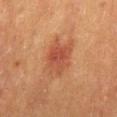| key | value |
|---|---|
| follow-up | catalogued during a skin exam; not biopsied |
| lighting | cross-polarized illumination |
| anatomic site | the mid back |
| patient | male, aged around 50 |
| image-analysis metrics | a footprint of about 14 mm², an eccentricity of roughly 0.75, and a shape-asymmetry score of about 0.25 (0 = symmetric); a border-irregularity index near 3/10, a color-variation rating of about 5/10, and peripheral color asymmetry of about 1.5; a nevus-likeness score of about 45/100 and a lesion-detection confidence of about 100/100 |
| size | ≈5 mm |
| acquisition | ~15 mm crop, total-body skin-cancer survey |